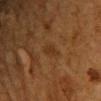The lesion was photographed on a routine skin check and not biopsied; there is no pathology result. Measured at roughly 3 mm in maximum diameter. From the chest. The subject is a male aged around 60. Imaged with cross-polarized lighting. A close-up tile cropped from a whole-body skin photograph, about 15 mm across.